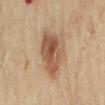Notes:
• follow-up — total-body-photography surveillance lesion; no biopsy
• automated lesion analysis — a lesion area of about 20 mm², an outline eccentricity of about 0.65 (0 = round, 1 = elongated), and a symmetry-axis asymmetry near 0.3
• image — ~15 mm crop, total-body skin-cancer survey
• body site — the mid back
• subject — female, aged approximately 60
• tile lighting — cross-polarized